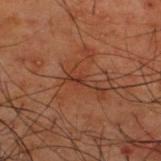| field | value |
|---|---|
| notes | catalogued during a skin exam; not biopsied |
| patient | male, aged approximately 50 |
| tile lighting | cross-polarized |
| diameter | ~3 mm (longest diameter) |
| acquisition | ~15 mm crop, total-body skin-cancer survey |
| TBP lesion metrics | an average lesion color of about L≈26 a*≈19 b*≈23 (CIELAB) and about 5 CIELAB-L* units darker than the surrounding skin; a border-irregularity index near 4.5/10, a within-lesion color-variation index near 0/10, and peripheral color asymmetry of about 0 |
| site | the upper back |